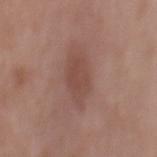• patient · male, aged 63 to 67
• lighting · white-light
• lesion size · about 5 mm
• acquisition · ~15 mm tile from a whole-body skin photo
• body site · the mid back